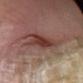Impression: The lesion was photographed on a routine skin check and not biopsied; there is no pathology result. Background: The subject is a male about 60 years old. The tile uses white-light illumination. The lesion is on the right lower leg. Cropped from a whole-body photographic skin survey; the tile spans about 15 mm. About 4.5 mm across.